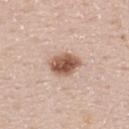The lesion was photographed on a routine skin check and not biopsied; there is no pathology result.
The lesion is located on the upper back.
A male subject approximately 40 years of age.
Captured under white-light illumination.
A roughly 15 mm field-of-view crop from a total-body skin photograph.